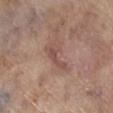Findings:
- notes: imaged on a skin check; not biopsied
- tile lighting: white-light illumination
- anatomic site: the right lower leg
- patient: female, roughly 85 years of age or older
- acquisition: 15 mm crop, total-body photography